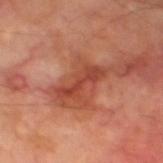Longest diameter approximately 5 mm.
A male subject, aged 68–72.
On the left thigh.
Cropped from a total-body skin-imaging series; the visible field is about 15 mm.
Imaged with cross-polarized lighting.